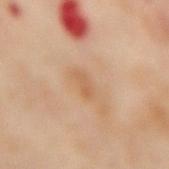workup: imaged on a skin check; not biopsied | site: the back | lighting: cross-polarized illumination | size: ≈2.5 mm | patient: female, aged 53 to 57 | image: 15 mm crop, total-body photography.